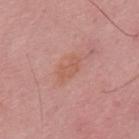Impression:
Recorded during total-body skin imaging; not selected for excision or biopsy.
Context:
This image is a 15 mm lesion crop taken from a total-body photograph. Captured under white-light illumination. On the mid back. The patient is a male aged approximately 50. Automated image analysis of the tile measured a normalized lesion–skin contrast near 5.5. Measured at roughly 3.5 mm in maximum diameter.